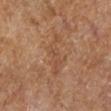This lesion was catalogued during total-body skin photography and was not selected for biopsy. A 15 mm close-up tile from a total-body photography series done for melanoma screening. This is a cross-polarized tile. About 5.5 mm across. The lesion is on the left lower leg. A female patient.